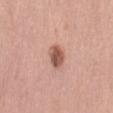| feature | finding |
|---|---|
| workup | no biopsy performed (imaged during a skin exam) |
| lighting | white-light illumination |
| site | the lower back |
| imaging modality | 15 mm crop, total-body photography |
| automated metrics | a footprint of about 5.5 mm², an eccentricity of roughly 0.75, and a symmetry-axis asymmetry near 0.25; an average lesion color of about L≈55 a*≈23 b*≈28 (CIELAB) and a normalized lesion–skin contrast near 9; a within-lesion color-variation index near 4/10 and a peripheral color-asymmetry measure near 1.5 |
| patient | female, in their 40s |
| lesion size | about 3 mm |